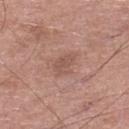Acquisition and patient details: The total-body-photography lesion software estimated an average lesion color of about L≈53 a*≈20 b*≈25 (CIELAB) and a normalized lesion–skin contrast near 5. The analysis additionally found a border-irregularity index near 3/10 and a peripheral color-asymmetry measure near 0.5. The patient is a male aged around 65. Approximately 3 mm at its widest. The lesion is located on the left lower leg. A region of skin cropped from a whole-body photographic capture, roughly 15 mm wide.